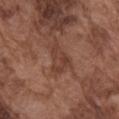Impression: Captured during whole-body skin photography for melanoma surveillance; the lesion was not biopsied. Background: A male patient aged 73 to 77. Cropped from a total-body skin-imaging series; the visible field is about 15 mm. The lesion-visualizer software estimated a border-irregularity index near 4.5/10, a within-lesion color-variation index near 3/10, and radial color variation of about 1. The software also gave a nevus-likeness score of about 0/100 and lesion-presence confidence of about 95/100. Located on the right upper arm. About 4.5 mm across.